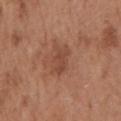Impression: This lesion was catalogued during total-body skin photography and was not selected for biopsy. Image and clinical context: A male patient aged around 65. Captured under white-light illumination. Approximately 3 mm at its widest. A close-up tile cropped from a whole-body skin photograph, about 15 mm across. On the mid back. Automated tile analysis of the lesion measured an average lesion color of about L≈46 a*≈23 b*≈30 (CIELAB) and a normalized lesion–skin contrast near 5.5. The analysis additionally found a border-irregularity rating of about 4.5/10, a color-variation rating of about 1/10, and radial color variation of about 0.5.